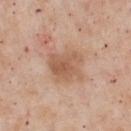Impression:
Imaged during a routine full-body skin examination; the lesion was not biopsied and no histopathology is available.
Background:
Automated image analysis of the tile measured a footprint of about 12 mm², a shape eccentricity near 0.5, and a symmetry-axis asymmetry near 0.35. And it measured a lesion color around L≈58 a*≈20 b*≈32 in CIELAB, a lesion–skin lightness drop of about 9, and a normalized border contrast of about 6.5. The analysis additionally found a border-irregularity rating of about 3.5/10. And it measured a classifier nevus-likeness of about 5/100 and a lesion-detection confidence of about 100/100. The patient is a male in their mid- to late 50s. The lesion is on the chest. A close-up tile cropped from a whole-body skin photograph, about 15 mm across. Longest diameter approximately 4 mm.